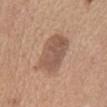This lesion was catalogued during total-body skin photography and was not selected for biopsy. A female subject, aged approximately 55. The lesion-visualizer software estimated an area of roughly 16 mm² and a symmetry-axis asymmetry near 0.15. The analysis additionally found about 10 CIELAB-L* units darker than the surrounding skin and a normalized border contrast of about 7.5. And it measured a lesion-detection confidence of about 100/100. The lesion is on the abdomen. The tile uses white-light illumination. A 15 mm crop from a total-body photograph taken for skin-cancer surveillance. The lesion's longest dimension is about 5.5 mm.